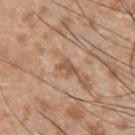{"biopsy_status": "not biopsied; imaged during a skin examination", "patient": {"sex": "male", "age_approx": 45}, "automated_metrics": {"eccentricity": 0.8, "shape_asymmetry": 0.45, "cielab_L": 54, "cielab_a": 20, "cielab_b": 32, "vs_skin_darker_L": 10.0, "vs_skin_contrast_norm": 7.0}, "lighting": "white-light", "lesion_size": {"long_diameter_mm_approx": 2.5}, "image": {"source": "total-body photography crop", "field_of_view_mm": 15}, "site": "upper back"}